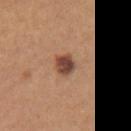{"lighting": "white-light", "site": "arm", "lesion_size": {"long_diameter_mm_approx": 3.0}, "patient": {"sex": "female", "age_approx": 25}, "image": {"source": "total-body photography crop", "field_of_view_mm": 15}, "automated_metrics": {"eccentricity": 0.65, "shape_asymmetry": 0.2, "border_irregularity_0_10": 2.0, "color_variation_0_10": 3.5, "peripheral_color_asymmetry": 1.0}}